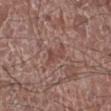Notes:
- follow-up — imaged on a skin check; not biopsied
- lesion size — ≈3.5 mm
- imaging modality — total-body-photography crop, ~15 mm field of view
- illumination — white-light
- patient — male, aged around 75
- anatomic site — the right lower leg